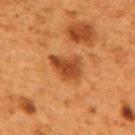Imaged during a routine full-body skin examination; the lesion was not biopsied and no histopathology is available. Longest diameter approximately 4.5 mm. Automated image analysis of the tile measured an area of roughly 8.5 mm², an outline eccentricity of about 0.75 (0 = round, 1 = elongated), and a symmetry-axis asymmetry near 0.35. It also reported a border-irregularity index near 3.5/10. The analysis additionally found a nevus-likeness score of about 80/100 and a detector confidence of about 100 out of 100 that the crop contains a lesion. On the upper back. A 15 mm close-up extracted from a 3D total-body photography capture. The patient is a female approximately 50 years of age.